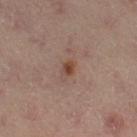No biopsy was performed on this lesion — it was imaged during a full skin examination and was not determined to be concerning.
The patient is a female aged 38 to 42.
A region of skin cropped from a whole-body photographic capture, roughly 15 mm wide.
Located on the right thigh.
The tile uses cross-polarized illumination.
Longest diameter approximately 1.5 mm.